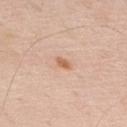Impression:
Imaged during a routine full-body skin examination; the lesion was not biopsied and no histopathology is available.